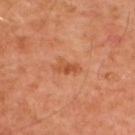Q: Was this lesion biopsied?
A: no biopsy performed (imaged during a skin exam)
Q: Lesion location?
A: the upper back
Q: Who is the patient?
A: male, in their 50s
Q: How was the tile lit?
A: cross-polarized
Q: What is the imaging modality?
A: 15 mm crop, total-body photography
Q: How large is the lesion?
A: ≈3.5 mm